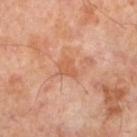No biopsy was performed on this lesion — it was imaged during a full skin examination and was not determined to be concerning.
On the left lower leg.
The total-body-photography lesion software estimated an average lesion color of about L≈58 a*≈25 b*≈35 (CIELAB) and a lesion–skin lightness drop of about 7. The software also gave a border-irregularity rating of about 4.5/10, internal color variation of about 1 on a 0–10 scale, and a peripheral color-asymmetry measure near 0.5.
A male patient, aged 48 to 52.
This image is a 15 mm lesion crop taken from a total-body photograph.
The tile uses cross-polarized illumination.